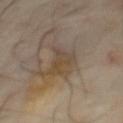<lesion>
<biopsy_status>not biopsied; imaged during a skin examination</biopsy_status>
<site>chest</site>
<patient>
  <sex>male</sex>
  <age_approx>65</age_approx>
</patient>
<lesion_size>
  <long_diameter_mm_approx>4.5</long_diameter_mm_approx>
</lesion_size>
<lighting>cross-polarized</lighting>
<image>
  <source>total-body photography crop</source>
  <field_of_view_mm>15</field_of_view_mm>
</image>
</lesion>